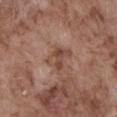Recorded during total-body skin imaging; not selected for excision or biopsy.
A roughly 15 mm field-of-view crop from a total-body skin photograph.
From the front of the torso.
The patient is a male aged around 75.